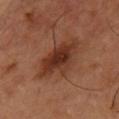Impression: The lesion was photographed on a routine skin check and not biopsied; there is no pathology result. Background: An algorithmic analysis of the crop reported a lesion area of about 17 mm² and two-axis asymmetry of about 0.25. The analysis additionally found a mean CIELAB color near L≈34 a*≈22 b*≈29, about 10 CIELAB-L* units darker than the surrounding skin, and a lesion-to-skin contrast of about 9 (normalized; higher = more distinct). The software also gave a border-irregularity rating of about 3.5/10 and a peripheral color-asymmetry measure near 1.5. The software also gave an automated nevus-likeness rating near 90 out of 100 and a detector confidence of about 100 out of 100 that the crop contains a lesion. This is a cross-polarized tile. A male subject, aged approximately 55. A 15 mm crop from a total-body photograph taken for skin-cancer surveillance. Approximately 7 mm at its widest. From the chest.